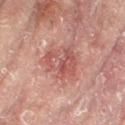Captured during whole-body skin photography for melanoma surveillance; the lesion was not biopsied. This is a cross-polarized tile. The lesion's longest dimension is about 5 mm. The lesion-visualizer software estimated a footprint of about 13 mm² and a shape eccentricity near 0.65. The software also gave an average lesion color of about L≈49 a*≈24 b*≈23 (CIELAB), a lesion–skin lightness drop of about 8, and a normalized lesion–skin contrast near 6. The analysis additionally found an automated nevus-likeness rating near 0 out of 100 and a lesion-detection confidence of about 100/100. The lesion is located on the left leg. The patient is a female aged around 80. A roughly 15 mm field-of-view crop from a total-body skin photograph.